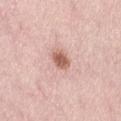subject = female, approximately 50 years of age
site = the back
image = total-body-photography crop, ~15 mm field of view